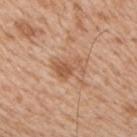biopsy status: total-body-photography surveillance lesion; no biopsy
lighting: white-light illumination
anatomic site: the right upper arm
subject: male, aged 63–67
image: 15 mm crop, total-body photography